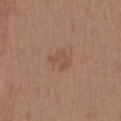No biopsy was performed on this lesion — it was imaged during a full skin examination and was not determined to be concerning.
The lesion is on the chest.
This image is a 15 mm lesion crop taken from a total-body photograph.
The recorded lesion diameter is about 2.5 mm.
Imaged with white-light lighting.
The patient is a male roughly 40 years of age.